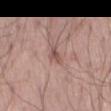Q: Was a biopsy performed?
A: no biopsy performed (imaged during a skin exam)
Q: How was the tile lit?
A: white-light illumination
Q: Patient demographics?
A: male, in their 70s
Q: What kind of image is this?
A: ~15 mm crop, total-body skin-cancer survey
Q: Lesion size?
A: ~3 mm (longest diameter)
Q: What is the anatomic site?
A: the left thigh